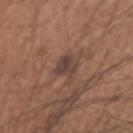Imaged during a routine full-body skin examination; the lesion was not biopsied and no histopathology is available. From the right upper arm. The patient is a male roughly 65 years of age. Cropped from a whole-body photographic skin survey; the tile spans about 15 mm. The total-body-photography lesion software estimated a border-irregularity rating of about 3/10, a color-variation rating of about 3.5/10, and radial color variation of about 1.5. The tile uses white-light illumination.